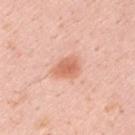Assessment:
Part of a total-body skin-imaging series; this lesion was reviewed on a skin check and was not flagged for biopsy.
Background:
Located on the arm. Longest diameter approximately 3.5 mm. The tile uses white-light illumination. A 15 mm close-up tile from a total-body photography series done for melanoma screening. The total-body-photography lesion software estimated a lesion area of about 6 mm² and a shape eccentricity near 0.75. The analysis additionally found a mean CIELAB color near L≈66 a*≈27 b*≈33, roughly 11 lightness units darker than nearby skin, and a normalized lesion–skin contrast near 7. The analysis additionally found a detector confidence of about 100 out of 100 that the crop contains a lesion. A male patient, aged around 35.